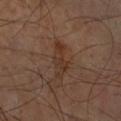- workup: imaged on a skin check; not biopsied
- patient: male, aged approximately 70
- lighting: cross-polarized illumination
- body site: the right forearm
- image: total-body-photography crop, ~15 mm field of view